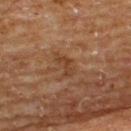{
  "biopsy_status": "not biopsied; imaged during a skin examination",
  "image": {
    "source": "total-body photography crop",
    "field_of_view_mm": 15
  },
  "lighting": "cross-polarized",
  "lesion_size": {
    "long_diameter_mm_approx": 3.0
  },
  "site": "upper back",
  "automated_metrics": {
    "area_mm2_approx": 4.0,
    "eccentricity": 0.85,
    "shape_asymmetry": 0.4,
    "cielab_L": 36,
    "cielab_a": 19,
    "cielab_b": 30,
    "vs_skin_darker_L": 6.0,
    "vs_skin_contrast_norm": 6.0
  },
  "patient": {
    "sex": "male",
    "age_approx": 65
  }
}